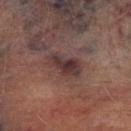Clinical impression:
The lesion was photographed on a routine skin check and not biopsied; there is no pathology result.
Clinical summary:
A lesion tile, about 15 mm wide, cut from a 3D total-body photograph. A male subject, aged around 75. Located on the left lower leg. An algorithmic analysis of the crop reported a classifier nevus-likeness of about 5/100 and a lesion-detection confidence of about 90/100. The lesion's longest dimension is about 4.5 mm.